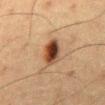Imaged during a routine full-body skin examination; the lesion was not biopsied and no histopathology is available. The lesion-visualizer software estimated a within-lesion color-variation index near 9/10. The tile uses cross-polarized illumination. Measured at roughly 3.5 mm in maximum diameter. The lesion is located on the mid back. A male subject roughly 60 years of age. A roughly 15 mm field-of-view crop from a total-body skin photograph.